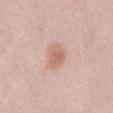Clinical impression: Imaged during a routine full-body skin examination; the lesion was not biopsied and no histopathology is available. Acquisition and patient details: The subject is a female aged around 60. Cropped from a total-body skin-imaging series; the visible field is about 15 mm. Imaged with white-light lighting. The lesion is located on the abdomen. Approximately 3 mm at its widest.